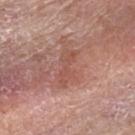biopsy status: imaged on a skin check; not biopsied | illumination: white-light | size: ~4.5 mm (longest diameter) | image: total-body-photography crop, ~15 mm field of view | anatomic site: the right forearm | subject: female, aged 43 to 47.